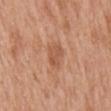<lesion>
<biopsy_status>not biopsied; imaged during a skin examination</biopsy_status>
<automated_metrics>
  <area_mm2_approx>5.0</area_mm2_approx>
  <eccentricity>0.65</eccentricity>
  <shape_asymmetry>0.25</shape_asymmetry>
  <cielab_L>55</cielab_L>
  <cielab_a>24</cielab_a>
  <cielab_b>34</cielab_b>
  <vs_skin_darker_L>8.0</vs_skin_darker_L>
  <vs_skin_contrast_norm>5.5</vs_skin_contrast_norm>
  <nevus_likeness_0_100>0</nevus_likeness_0_100>
  <lesion_detection_confidence_0_100>100</lesion_detection_confidence_0_100>
</automated_metrics>
<patient>
  <sex>female</sex>
  <age_approx>40</age_approx>
</patient>
<site>mid back</site>
<image>
  <source>total-body photography crop</source>
  <field_of_view_mm>15</field_of_view_mm>
</image>
<lesion_size>
  <long_diameter_mm_approx>3.0</long_diameter_mm_approx>
</lesion_size>
</lesion>